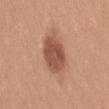  biopsy_status: not biopsied; imaged during a skin examination
  image:
    source: total-body photography crop
    field_of_view_mm: 15
  site: mid back
  patient:
    sex: female
    age_approx: 35
  lesion_size:
    long_diameter_mm_approx: 5.5
  automated_metrics:
    area_mm2_approx: 13.0
    eccentricity: 0.85
    shape_asymmetry: 0.15
    cielab_L: 52
    cielab_a: 23
    cielab_b: 29
    border_irregularity_0_10: 1.5
    color_variation_0_10: 3.0
    peripheral_color_asymmetry: 1.0
    nevus_likeness_0_100: 95
    lesion_detection_confidence_0_100: 100
  lighting: white-light